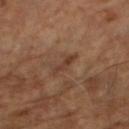  biopsy_status: not biopsied; imaged during a skin examination